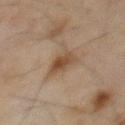Clinical impression:
The lesion was tiled from a total-body skin photograph and was not biopsied.
Background:
The lesion is located on the chest. The tile uses cross-polarized illumination. A male subject aged around 70. The lesion-visualizer software estimated an area of roughly 6 mm², a shape eccentricity near 0.75, and two-axis asymmetry of about 0.25. And it measured a mean CIELAB color near L≈38 a*≈14 b*≈26 and a normalized border contrast of about 8. About 3.5 mm across. A roughly 15 mm field-of-view crop from a total-body skin photograph.